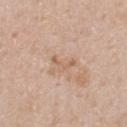  biopsy_status: not biopsied; imaged during a skin examination
  site: upper back
  lighting: white-light
  lesion_size:
    long_diameter_mm_approx: 3.0
  image:
    source: total-body photography crop
    field_of_view_mm: 15
  automated_metrics:
    cielab_L: 61
    cielab_a: 18
    cielab_b: 31
    border_irregularity_0_10: 8.0
    color_variation_0_10: 0.0
    peripheral_color_asymmetry: 0.0
    nevus_likeness_0_100: 0
    lesion_detection_confidence_0_100: 100
  patient:
    sex: male
    age_approx: 40